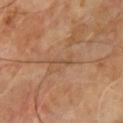No biopsy was performed on this lesion — it was imaged during a full skin examination and was not determined to be concerning. On the front of the torso. Measured at roughly 3 mm in maximum diameter. The tile uses cross-polarized illumination. The patient is a male in their mid- to late 60s. This image is a 15 mm lesion crop taken from a total-body photograph.